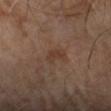– body site: the left forearm
– lighting: cross-polarized illumination
– subject: male, aged 63–67
– automated metrics: an area of roughly 3 mm², an eccentricity of roughly 0.85, and a symmetry-axis asymmetry near 0.45
– imaging modality: 15 mm crop, total-body photography
– lesion diameter: ≈2.5 mm